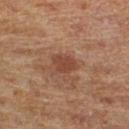<tbp_lesion>
  <biopsy_status>not biopsied; imaged during a skin examination</biopsy_status>
  <lesion_size>
    <long_diameter_mm_approx>3.5</long_diameter_mm_approx>
  </lesion_size>
  <image>
    <source>total-body photography crop</source>
    <field_of_view_mm>15</field_of_view_mm>
  </image>
  <lighting>cross-polarized</lighting>
  <site>right lower leg</site>
  <patient>
    <sex>male</sex>
    <age_approx>65</age_approx>
  </patient>
  <automated_metrics>
    <cielab_L>39</cielab_L>
    <cielab_a>20</cielab_a>
    <cielab_b>26</cielab_b>
    <vs_skin_darker_L>8.0</vs_skin_darker_L>
    <vs_skin_contrast_norm>6.5</vs_skin_contrast_norm>
    <border_irregularity_0_10>3.0</border_irregularity_0_10>
    <color_variation_0_10>1.5</color_variation_0_10>
    <peripheral_color_asymmetry>0.5</peripheral_color_asymmetry>
    <nevus_likeness_0_100>5</nevus_likeness_0_100>
    <lesion_detection_confidence_0_100>100</lesion_detection_confidence_0_100>
  </automated_metrics>
</tbp_lesion>